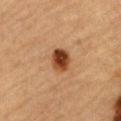notes: total-body-photography surveillance lesion; no biopsy | imaging modality: ~15 mm tile from a whole-body skin photo | patient: male, in their mid- to late 80s or older | site: the abdomen.